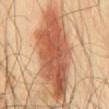follow-up: total-body-photography surveillance lesion; no biopsy | lighting: cross-polarized | patient: male, aged 48–52 | image source: ~15 mm crop, total-body skin-cancer survey | lesion diameter: about 11.5 mm | automated lesion analysis: a lesion–skin lightness drop of about 16 and a normalized lesion–skin contrast near 10 | site: the mid back.